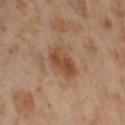Part of a total-body skin-imaging series; this lesion was reviewed on a skin check and was not flagged for biopsy. The lesion is located on the right thigh. The subject is a female in their mid-50s. Captured under cross-polarized illumination. This image is a 15 mm lesion crop taken from a total-body photograph.